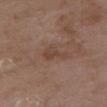Recorded during total-body skin imaging; not selected for excision or biopsy. A 15 mm close-up extracted from a 3D total-body photography capture. The patient is a female aged around 70. Longest diameter approximately 3.5 mm. Imaged with white-light lighting. On the upper back.